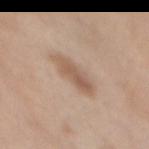workup — total-body-photography surveillance lesion; no biopsy | body site — the chest | patient — female, aged 63 to 67 | size — ~5.5 mm (longest diameter) | lighting — white-light illumination | imaging modality — ~15 mm crop, total-body skin-cancer survey.